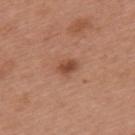acquisition: ~15 mm crop, total-body skin-cancer survey
subject: female, roughly 40 years of age
lighting: white-light illumination
TBP lesion metrics: an outline eccentricity of about 0.7 (0 = round, 1 = elongated) and two-axis asymmetry of about 0.25; a lesion color around L≈48 a*≈24 b*≈32 in CIELAB, about 10 CIELAB-L* units darker than the surrounding skin, and a lesion-to-skin contrast of about 8 (normalized; higher = more distinct); border irregularity of about 2.5 on a 0–10 scale and a color-variation rating of about 2/10
body site: the upper back